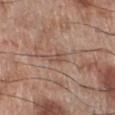biopsy status = catalogued during a skin exam; not biopsied
subject = male, roughly 70 years of age
anatomic site = the right lower leg
image source = ~15 mm crop, total-body skin-cancer survey